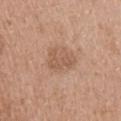Findings:
- image source · ~15 mm crop, total-body skin-cancer survey
- diameter · ≈3.5 mm
- lighting · white-light
- patient · male, aged 38–42
- site · the upper back